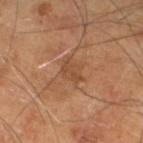Clinical impression: Part of a total-body skin-imaging series; this lesion was reviewed on a skin check and was not flagged for biopsy. Background: Imaged with cross-polarized lighting. About 3 mm across. The patient is a male approximately 65 years of age. Automated tile analysis of the lesion measured an eccentricity of roughly 0.75 and two-axis asymmetry of about 0.25. A region of skin cropped from a whole-body photographic capture, roughly 15 mm wide. From the left lower leg.